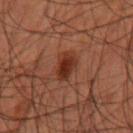Q: Is there a histopathology result?
A: no biopsy performed (imaged during a skin exam)
Q: How was the tile lit?
A: cross-polarized illumination
Q: Automated lesion metrics?
A: a nevus-likeness score of about 95/100 and lesion-presence confidence of about 100/100
Q: Lesion location?
A: the right forearm
Q: Patient demographics?
A: male, aged around 60
Q: What kind of image is this?
A: ~15 mm tile from a whole-body skin photo
Q: What is the lesion's diameter?
A: about 3 mm